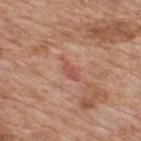Part of a total-body skin-imaging series; this lesion was reviewed on a skin check and was not flagged for biopsy. This is a white-light tile. A 15 mm close-up tile from a total-body photography series done for melanoma screening. The lesion is on the back. A male patient in their 60s. An algorithmic analysis of the crop reported an area of roughly 3.5 mm². The recorded lesion diameter is about 3 mm.